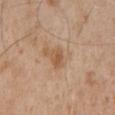{
  "biopsy_status": "not biopsied; imaged during a skin examination",
  "lighting": "white-light",
  "image": {
    "source": "total-body photography crop",
    "field_of_view_mm": 15
  },
  "site": "chest",
  "lesion_size": {
    "long_diameter_mm_approx": 2.5
  },
  "patient": {
    "sex": "male",
    "age_approx": 65
  },
  "automated_metrics": {
    "area_mm2_approx": 3.5,
    "shape_asymmetry": 0.25,
    "vs_skin_darker_L": 8.0,
    "vs_skin_contrast_norm": 6.5,
    "border_irregularity_0_10": 2.5,
    "peripheral_color_asymmetry": 1.0
  }
}